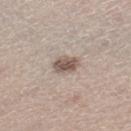A female subject, approximately 40 years of age. Located on the right lower leg. A 15 mm close-up tile from a total-body photography series done for melanoma screening.